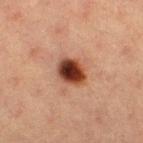Findings:
• biopsy status: no biopsy performed (imaged during a skin exam)
• TBP lesion metrics: a lesion area of about 8.5 mm², an outline eccentricity of about 0.55 (0 = round, 1 = elongated), and a shape-asymmetry score of about 0.2 (0 = symmetric); a nevus-likeness score of about 100/100 and a detector confidence of about 100 out of 100 that the crop contains a lesion
• body site: the right thigh
• lesion diameter: about 3 mm
• imaging modality: total-body-photography crop, ~15 mm field of view
• patient: female, aged approximately 40